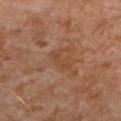This lesion was catalogued during total-body skin photography and was not selected for biopsy.
Automated tile analysis of the lesion measured a shape eccentricity near 0.95 and a shape-asymmetry score of about 0.35 (0 = symmetric). And it measured an average lesion color of about L≈44 a*≈21 b*≈31 (CIELAB), a lesion–skin lightness drop of about 6, and a lesion-to-skin contrast of about 5 (normalized; higher = more distinct). And it measured a border-irregularity rating of about 5/10 and a within-lesion color-variation index near 1/10.
A 15 mm close-up tile from a total-body photography series done for melanoma screening.
A male subject aged 28–32.
On the left lower leg.